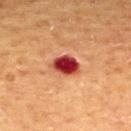Case summary:
• workup: imaged on a skin check; not biopsied
• anatomic site: the upper back
• image: total-body-photography crop, ~15 mm field of view
• tile lighting: cross-polarized
• image-analysis metrics: a border-irregularity rating of about 2/10, internal color variation of about 5 on a 0–10 scale, and radial color variation of about 1.5; a classifier nevus-likeness of about 0/100
• lesion size: ~3.5 mm (longest diameter)
• patient: male, about 60 years old